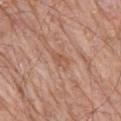Clinical summary:
Cropped from a whole-body photographic skin survey; the tile spans about 15 mm. The lesion is located on the back. The recorded lesion diameter is about 2.5 mm. The patient is a male aged 78 to 82.